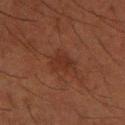Q: Was a biopsy performed?
A: catalogued during a skin exam; not biopsied
Q: Who is the patient?
A: male, aged 58–62
Q: What lighting was used for the tile?
A: cross-polarized illumination
Q: Lesion location?
A: the right forearm
Q: How was this image acquired?
A: total-body-photography crop, ~15 mm field of view